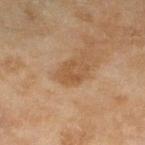<tbp_lesion>
<biopsy_status>not biopsied; imaged during a skin examination</biopsy_status>
<lesion_size>
  <long_diameter_mm_approx>3.5</long_diameter_mm_approx>
</lesion_size>
<lighting>cross-polarized</lighting>
<image>
  <source>total-body photography crop</source>
  <field_of_view_mm>15</field_of_view_mm>
</image>
<automated_metrics>
  <cielab_L>48</cielab_L>
  <cielab_a>18</cielab_a>
  <cielab_b>33</cielab_b>
  <vs_skin_darker_L>7.0</vs_skin_darker_L>
  <nevus_likeness_0_100>0</nevus_likeness_0_100>
  <lesion_detection_confidence_0_100>100</lesion_detection_confidence_0_100>
</automated_metrics>
<site>leg</site>
<patient>
  <sex>female</sex>
  <age_approx>60</age_approx>
</patient>
</tbp_lesion>